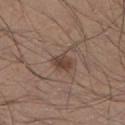<tbp_lesion>
  <biopsy_status>not biopsied; imaged during a skin examination</biopsy_status>
  <site>right lower leg</site>
  <lesion_size>
    <long_diameter_mm_approx>2.5</long_diameter_mm_approx>
  </lesion_size>
  <patient>
    <sex>male</sex>
    <age_approx>35</age_approx>
  </patient>
  <image>
    <source>total-body photography crop</source>
    <field_of_view_mm>15</field_of_view_mm>
  </image>
  <lighting>white-light</lighting>
</tbp_lesion>